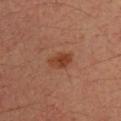The lesion was tiled from a total-body skin photograph and was not biopsied.
This is a cross-polarized tile.
Located on the arm.
A 15 mm close-up tile from a total-body photography series done for melanoma screening.
Measured at roughly 3 mm in maximum diameter.
The total-body-photography lesion software estimated a lesion–skin lightness drop of about 8 and a lesion-to-skin contrast of about 8 (normalized; higher = more distinct).
A male subject, roughly 35 years of age.